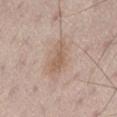From the lower back. A male subject aged 63–67. A lesion tile, about 15 mm wide, cut from a 3D total-body photograph. Imaged with white-light lighting. Longest diameter approximately 4 mm.